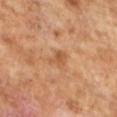lighting: cross-polarized | subject: male, roughly 65 years of age | image-analysis metrics: a lesion area of about 3 mm², an outline eccentricity of about 0.8 (0 = round, 1 = elongated), and two-axis asymmetry of about 0.35; about 8 CIELAB-L* units darker than the surrounding skin and a normalized border contrast of about 6 | lesion diameter: ≈2.5 mm | acquisition: ~15 mm crop, total-body skin-cancer survey.